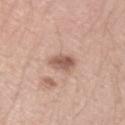Assessment: No biopsy was performed on this lesion — it was imaged during a full skin examination and was not determined to be concerning. Background: Imaged with white-light lighting. Automated image analysis of the tile measured an area of roughly 5.5 mm², a shape eccentricity near 0.5, and a shape-asymmetry score of about 0.25 (0 = symmetric). The software also gave a normalized lesion–skin contrast near 8. And it measured border irregularity of about 2.5 on a 0–10 scale. The lesion is on the right upper arm. The subject is a male aged 28–32. Longest diameter approximately 3 mm. This image is a 15 mm lesion crop taken from a total-body photograph.